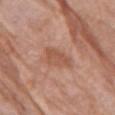From the right upper arm. The total-body-photography lesion software estimated a mean CIELAB color near L≈53 a*≈23 b*≈31, a lesion–skin lightness drop of about 8, and a normalized border contrast of about 6. It also reported a border-irregularity rating of about 3.5/10 and a peripheral color-asymmetry measure near 0.5. It also reported an automated nevus-likeness rating near 0 out of 100 and lesion-presence confidence of about 100/100. The tile uses white-light illumination. A female patient aged around 85 or older. A region of skin cropped from a whole-body photographic capture, roughly 15 mm wide. The recorded lesion diameter is about 3.5 mm.